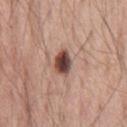| key | value |
|---|---|
| follow-up | no biopsy performed (imaged during a skin exam) |
| TBP lesion metrics | a nevus-likeness score of about 100/100 and a lesion-detection confidence of about 100/100 |
| illumination | white-light illumination |
| lesion diameter | about 3 mm |
| patient | male, aged 53 to 57 |
| image source | ~15 mm crop, total-body skin-cancer survey |
| site | the front of the torso |